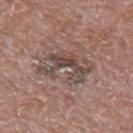{"biopsy_status": "not biopsied; imaged during a skin examination", "image": {"source": "total-body photography crop", "field_of_view_mm": 15}, "lesion_size": {"long_diameter_mm_approx": 6.0}, "patient": {"sex": "male", "age_approx": 70}, "lighting": "white-light", "site": "mid back", "automated_metrics": {"nevus_likeness_0_100": 0, "lesion_detection_confidence_0_100": 65}}